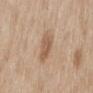The lesion was tiled from a total-body skin photograph and was not biopsied. A region of skin cropped from a whole-body photographic capture, roughly 15 mm wide. A female patient, aged approximately 60. Automated image analysis of the tile measured a footprint of about 6.5 mm² and a symmetry-axis asymmetry near 0.2. The software also gave a detector confidence of about 100 out of 100 that the crop contains a lesion. On the back. Approximately 3.5 mm at its widest.